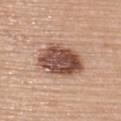Part of a total-body skin-imaging series; this lesion was reviewed on a skin check and was not flagged for biopsy. A roughly 15 mm field-of-view crop from a total-body skin photograph. The lesion is on the upper back. The total-body-photography lesion software estimated an outline eccentricity of about 0.7 (0 = round, 1 = elongated). The software also gave a mean CIELAB color near L≈50 a*≈20 b*≈27 and a lesion–skin lightness drop of about 17. The software also gave an automated nevus-likeness rating near 20 out of 100 and a detector confidence of about 100 out of 100 that the crop contains a lesion. A female subject aged approximately 65. The recorded lesion diameter is about 5.5 mm. This is a white-light tile.